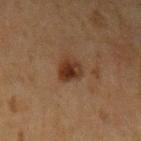Assessment:
Recorded during total-body skin imaging; not selected for excision or biopsy.
Clinical summary:
The lesion-visualizer software estimated about 10 CIELAB-L* units darker than the surrounding skin and a normalized lesion–skin contrast near 10. A roughly 15 mm field-of-view crop from a total-body skin photograph. The lesion is located on the left upper arm. The tile uses cross-polarized illumination. A male subject aged approximately 50. Longest diameter approximately 3 mm.